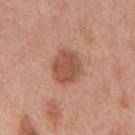No biopsy was performed on this lesion — it was imaged during a full skin examination and was not determined to be concerning. A close-up tile cropped from a whole-body skin photograph, about 15 mm across. The subject is a male about 70 years old. The total-body-photography lesion software estimated a shape eccentricity near 0.45. The software also gave a border-irregularity rating of about 1.5/10, a within-lesion color-variation index near 2/10, and radial color variation of about 1. The software also gave a nevus-likeness score of about 25/100 and lesion-presence confidence of about 100/100. On the back. This is a white-light tile.